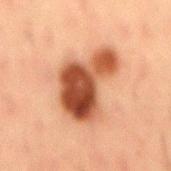| key | value |
|---|---|
| follow-up | total-body-photography surveillance lesion; no biopsy |
| image source | ~15 mm crop, total-body skin-cancer survey |
| patient | male, approximately 50 years of age |
| diameter | about 7.5 mm |
| location | the mid back |
| tile lighting | cross-polarized |